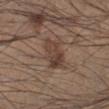Part of a total-body skin-imaging series; this lesion was reviewed on a skin check and was not flagged for biopsy.
Located on the left lower leg.
Captured under white-light illumination.
A male patient, in their mid- to late 30s.
Measured at roughly 4.5 mm in maximum diameter.
The total-body-photography lesion software estimated a within-lesion color-variation index near 5.5/10 and a peripheral color-asymmetry measure near 2. And it measured a classifier nevus-likeness of about 25/100 and lesion-presence confidence of about 100/100.
A 15 mm crop from a total-body photograph taken for skin-cancer surveillance.